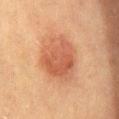workup: total-body-photography surveillance lesion; no biopsy | acquisition: 15 mm crop, total-body photography | site: the mid back | patient: female, roughly 40 years of age | illumination: cross-polarized | lesion size: ~6 mm (longest diameter).